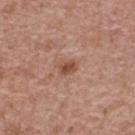| key | value |
|---|---|
| follow-up | catalogued during a skin exam; not biopsied |
| imaging modality | ~15 mm tile from a whole-body skin photo |
| tile lighting | white-light |
| patient | female, aged approximately 65 |
| anatomic site | the upper back |